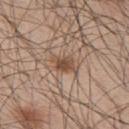- follow-up — no biopsy performed (imaged during a skin exam)
- lighting — white-light
- lesion diameter — ≈3.5 mm
- image source — total-body-photography crop, ~15 mm field of view
- subject — male, in their mid-40s
- location — the upper back